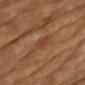<case>
  <biopsy_status>not biopsied; imaged during a skin examination</biopsy_status>
  <lighting>cross-polarized</lighting>
  <image>
    <source>total-body photography crop</source>
    <field_of_view_mm>15</field_of_view_mm>
  </image>
  <site>chest</site>
</case>